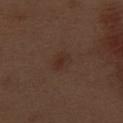Captured during whole-body skin photography for melanoma surveillance; the lesion was not biopsied. The lesion is on the right thigh. The tile uses white-light illumination. The subject is a male in their 70s. The lesion's longest dimension is about 2.5 mm. A close-up tile cropped from a whole-body skin photograph, about 15 mm across.